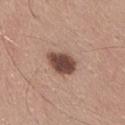Clinical impression:
Captured during whole-body skin photography for melanoma surveillance; the lesion was not biopsied.
Image and clinical context:
About 4 mm across. The tile uses white-light illumination. The subject is a male in their mid- to late 20s. The lesion is located on the chest. A lesion tile, about 15 mm wide, cut from a 3D total-body photograph.